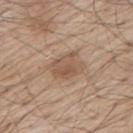Recorded during total-body skin imaging; not selected for excision or biopsy. Cropped from a total-body skin-imaging series; the visible field is about 15 mm. The lesion is on the mid back. The tile uses white-light illumination. The lesion's longest dimension is about 4 mm. The total-body-photography lesion software estimated an eccentricity of roughly 0.7 and two-axis asymmetry of about 0.2. And it measured an average lesion color of about L≈54 a*≈17 b*≈29 (CIELAB) and a normalized border contrast of about 6. The software also gave a border-irregularity rating of about 2.5/10 and a peripheral color-asymmetry measure near 1.5. The software also gave a nevus-likeness score of about 25/100 and a detector confidence of about 100 out of 100 that the crop contains a lesion. The subject is a male aged approximately 70.